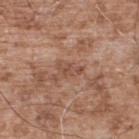Recorded during total-body skin imaging; not selected for excision or biopsy. A male subject aged 53 to 57. Located on the upper back. The recorded lesion diameter is about 3 mm. Automated tile analysis of the lesion measured a footprint of about 3 mm² and a symmetry-axis asymmetry near 0.45. The software also gave roughly 7 lightness units darker than nearby skin and a lesion-to-skin contrast of about 5.5 (normalized; higher = more distinct). And it measured a lesion-detection confidence of about 95/100. A close-up tile cropped from a whole-body skin photograph, about 15 mm across. The tile uses white-light illumination.